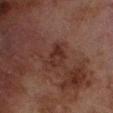Imaged during a routine full-body skin examination; the lesion was not biopsied and no histopathology is available. A male subject in their 70s. Approximately 3 mm at its widest. The total-body-photography lesion software estimated a lesion area of about 5 mm² and an outline eccentricity of about 0.75 (0 = round, 1 = elongated). The software also gave a mean CIELAB color near L≈24 a*≈17 b*≈20, a lesion–skin lightness drop of about 6, and a lesion-to-skin contrast of about 7 (normalized; higher = more distinct). And it measured a border-irregularity rating of about 2/10, a within-lesion color-variation index near 3.5/10, and a peripheral color-asymmetry measure near 1.5. Located on the left lower leg. Imaged with cross-polarized lighting. Cropped from a total-body skin-imaging series; the visible field is about 15 mm.